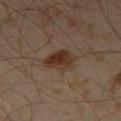Impression:
Imaged during a routine full-body skin examination; the lesion was not biopsied and no histopathology is available.
Context:
From the left thigh. Cropped from a total-body skin-imaging series; the visible field is about 15 mm. A male subject aged 43–47.